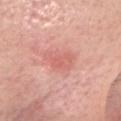A region of skin cropped from a whole-body photographic capture, roughly 15 mm wide.
The lesion's longest dimension is about 3.5 mm.
On the head or neck.
A female patient aged approximately 70.
This is a white-light tile.
Automated tile analysis of the lesion measured a lesion area of about 6.5 mm², a shape eccentricity near 0.75, and a shape-asymmetry score of about 0.35 (0 = symmetric). It also reported a border-irregularity index near 3.5/10 and internal color variation of about 2.5 on a 0–10 scale. It also reported a classifier nevus-likeness of about 5/100 and lesion-presence confidence of about 100/100.
On excision, pathology confirmed an actinic keratosis, classified as an indeterminate (borderline) lesion.